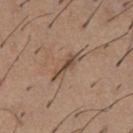biopsy status — catalogued during a skin exam; not biopsied | subject — male, aged around 60 | diameter — ~4 mm (longest diameter) | image — ~15 mm crop, total-body skin-cancer survey | body site — the front of the torso.